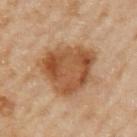* workup — no biopsy performed (imaged during a skin exam)
* imaging modality — total-body-photography crop, ~15 mm field of view
* image-analysis metrics — a lesion color around L≈52 a*≈22 b*≈36 in CIELAB, about 12 CIELAB-L* units darker than the surrounding skin, and a normalized border contrast of about 9; internal color variation of about 5.5 on a 0–10 scale and radial color variation of about 1.5
* subject — female, aged around 60
* location — the left upper arm